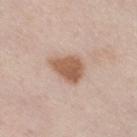biopsy_status: not biopsied; imaged during a skin examination
patient:
  sex: male
  age_approx: 60
lesion_size:
  long_diameter_mm_approx: 4.0
automated_metrics:
  area_mm2_approx: 9.5
  eccentricity: 0.7
  cielab_L: 58
  cielab_a: 19
  cielab_b: 30
  vs_skin_contrast_norm: 9.0
lighting: white-light
site: right thigh
image:
  source: total-body photography crop
  field_of_view_mm: 15